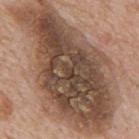notes=no biopsy performed (imaged during a skin exam); illumination=white-light illumination; imaging modality=~15 mm tile from a whole-body skin photo; anatomic site=the mid back; patient=male, roughly 65 years of age.